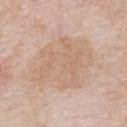This lesion was catalogued during total-body skin photography and was not selected for biopsy. A male subject in their 80s. A region of skin cropped from a whole-body photographic capture, roughly 15 mm wide. Measured at roughly 7.5 mm in maximum diameter. Captured under white-light illumination. Located on the front of the torso. The lesion-visualizer software estimated a footprint of about 33 mm², an eccentricity of roughly 0.6, and two-axis asymmetry of about 0.2. The analysis additionally found an average lesion color of about L≈66 a*≈16 b*≈29 (CIELAB) and about 6 CIELAB-L* units darker than the surrounding skin. It also reported internal color variation of about 3 on a 0–10 scale and radial color variation of about 1. It also reported a classifier nevus-likeness of about 0/100 and lesion-presence confidence of about 100/100.